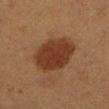This lesion was catalogued during total-body skin photography and was not selected for biopsy.